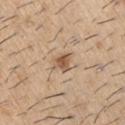Findings:
* notes — total-body-photography surveillance lesion; no biopsy
* image-analysis metrics — an area of roughly 4.5 mm², an eccentricity of roughly 0.5, and a symmetry-axis asymmetry near 0.4; a border-irregularity rating of about 3.5/10 and peripheral color asymmetry of about 1
* location — the left upper arm
* lighting — white-light illumination
* patient — male, aged 58–62
* lesion size — about 3 mm
* imaging modality — total-body-photography crop, ~15 mm field of view